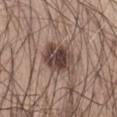Clinical summary: The recorded lesion diameter is about 5 mm. Automated image analysis of the tile measured a lesion color around L≈43 a*≈16 b*≈21 in CIELAB and about 14 CIELAB-L* units darker than the surrounding skin. And it measured an automated nevus-likeness rating near 70 out of 100. The patient is a male aged 38 to 42. A roughly 15 mm field-of-view crop from a total-body skin photograph. This is a white-light tile. From the abdomen.